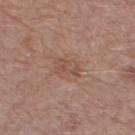Assessment: The lesion was tiled from a total-body skin photograph and was not biopsied. Background: Imaged with white-light lighting. The lesion is located on the right thigh. A female subject, roughly 55 years of age. A 15 mm crop from a total-body photograph taken for skin-cancer surveillance. The recorded lesion diameter is about 3.5 mm. The lesion-visualizer software estimated an area of roughly 4.5 mm², a shape eccentricity near 0.85, and a shape-asymmetry score of about 0.35 (0 = symmetric). It also reported lesion-presence confidence of about 100/100.